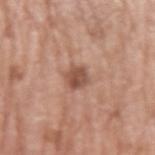Q: Was this lesion biopsied?
A: imaged on a skin check; not biopsied